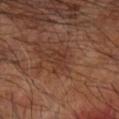The lesion was photographed on a routine skin check and not biopsied; there is no pathology result. A male subject aged around 65. Approximately 3 mm at its widest. From the right forearm. This is a cross-polarized tile. A 15 mm close-up tile from a total-body photography series done for melanoma screening.